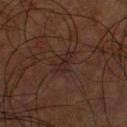This lesion was catalogued during total-body skin photography and was not selected for biopsy. A male subject aged approximately 60. The lesion is on the chest. The recorded lesion diameter is about 3.5 mm. The tile uses cross-polarized illumination. Cropped from a total-body skin-imaging series; the visible field is about 15 mm.